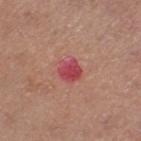follow-up: catalogued during a skin exam; not biopsied
acquisition: 15 mm crop, total-body photography
patient: female, in their 40s
illumination: white-light
location: the left thigh
diameter: ≈2.5 mm